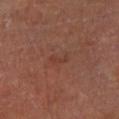Captured during whole-body skin photography for melanoma surveillance; the lesion was not biopsied. Imaged with cross-polarized lighting. Cropped from a whole-body photographic skin survey; the tile spans about 15 mm. Automated tile analysis of the lesion measured a shape eccentricity near 0.95 and a symmetry-axis asymmetry near 0.55. It also reported a mean CIELAB color near L≈35 a*≈22 b*≈26, about 5 CIELAB-L* units darker than the surrounding skin, and a lesion-to-skin contrast of about 4.5 (normalized; higher = more distinct). On the left lower leg. The recorded lesion diameter is about 2.5 mm. A male patient, aged approximately 65.